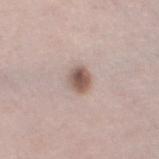Case summary:
– image-analysis metrics · a footprint of about 5.5 mm², a shape eccentricity near 0.5, and a shape-asymmetry score of about 0.2 (0 = symmetric); a border-irregularity rating of about 1.5/10, internal color variation of about 4.5 on a 0–10 scale, and radial color variation of about 1.5
– tile lighting · white-light
– anatomic site · the left thigh
– size · ~3 mm (longest diameter)
– image · ~15 mm crop, total-body skin-cancer survey
– patient · male, in their mid-60s